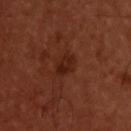Q: Was this lesion biopsied?
A: total-body-photography surveillance lesion; no biopsy
Q: What are the patient's age and sex?
A: male, aged 53–57
Q: What kind of image is this?
A: total-body-photography crop, ~15 mm field of view
Q: Where on the body is the lesion?
A: the upper back